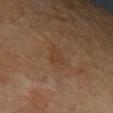| feature | finding |
|---|---|
| notes | catalogued during a skin exam; not biopsied |
| subject | female, aged 53–57 |
| acquisition | ~15 mm crop, total-body skin-cancer survey |
| size | about 3 mm |
| body site | the left forearm |
| illumination | cross-polarized |